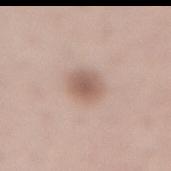This lesion was catalogued during total-body skin photography and was not selected for biopsy. About 3 mm across. This is a white-light tile. The lesion-visualizer software estimated an area of roughly 6.5 mm², a shape eccentricity near 0.4, and two-axis asymmetry of about 0.1. It also reported a lesion color around L≈58 a*≈18 b*≈25 in CIELAB and roughly 11 lightness units darker than nearby skin. The analysis additionally found a border-irregularity rating of about 1/10 and radial color variation of about 1. The software also gave a nevus-likeness score of about 85/100 and a lesion-detection confidence of about 100/100. A roughly 15 mm field-of-view crop from a total-body skin photograph. A male patient, in their mid- to late 50s. On the lower back.